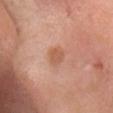size: ≈2.5 mm | site: the head or neck | patient: female, aged 28 to 32 | image source: 15 mm crop, total-body photography.